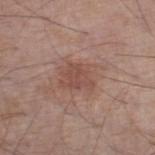* notes · catalogued during a skin exam; not biopsied